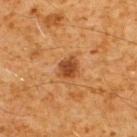The lesion was tiled from a total-body skin photograph and was not biopsied. An algorithmic analysis of the crop reported a lesion area of about 5 mm², an eccentricity of roughly 0.5, and a symmetry-axis asymmetry near 0.25. The software also gave a mean CIELAB color near L≈39 a*≈22 b*≈34, a lesion–skin lightness drop of about 11, and a normalized border contrast of about 9. The software also gave a border-irregularity index near 2/10 and radial color variation of about 1. Longest diameter approximately 2.5 mm. Captured under cross-polarized illumination. A male subject, aged 58 to 62. The lesion is located on the upper back. A 15 mm close-up extracted from a 3D total-body photography capture.